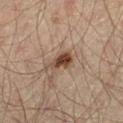site: the leg | subject: male, aged around 45 | image source: ~15 mm tile from a whole-body skin photo | automated lesion analysis: a lesion area of about 5 mm², a shape eccentricity near 0.7, and a shape-asymmetry score of about 0.3 (0 = symmetric); a border-irregularity index near 3/10, internal color variation of about 3 on a 0–10 scale, and radial color variation of about 1; a nevus-likeness score of about 95/100 and a detector confidence of about 100 out of 100 that the crop contains a lesion | lesion size: ≈3 mm | lighting: cross-polarized.